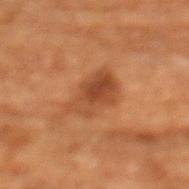Recorded during total-body skin imaging; not selected for excision or biopsy.
Imaged with cross-polarized lighting.
Cropped from a total-body skin-imaging series; the visible field is about 15 mm.
About 5 mm across.
On the front of the torso.
A female patient, approximately 80 years of age.